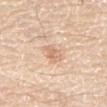The lesion was tiled from a total-body skin photograph and was not biopsied.
The lesion is located on the front of the torso.
The subject is a male approximately 80 years of age.
Cropped from a total-body skin-imaging series; the visible field is about 15 mm.
About 2.5 mm across.
The total-body-photography lesion software estimated an area of roughly 4.5 mm², a shape eccentricity near 0.75, and a shape-asymmetry score of about 0.25 (0 = symmetric). The analysis additionally found a mean CIELAB color near L≈69 a*≈19 b*≈33, roughly 9 lightness units darker than nearby skin, and a normalized border contrast of about 5.5. It also reported a border-irregularity index near 3/10, internal color variation of about 2 on a 0–10 scale, and peripheral color asymmetry of about 1. The software also gave an automated nevus-likeness rating near 0 out of 100 and lesion-presence confidence of about 100/100.
Imaged with white-light lighting.